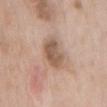Clinical impression: Captured during whole-body skin photography for melanoma surveillance; the lesion was not biopsied. Context: The lesion is on the mid back. A close-up tile cropped from a whole-body skin photograph, about 15 mm across. Captured under white-light illumination. A male subject aged approximately 65. Longest diameter approximately 4.5 mm. The total-body-photography lesion software estimated an area of roughly 9 mm², an eccentricity of roughly 0.85, and a shape-asymmetry score of about 0.2 (0 = symmetric). And it measured a color-variation rating of about 3.5/10 and radial color variation of about 1. The software also gave lesion-presence confidence of about 100/100.